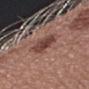Captured during whole-body skin photography for melanoma surveillance; the lesion was not biopsied.
A 15 mm crop from a total-body photograph taken for skin-cancer surveillance.
The subject is a female approximately 50 years of age.
On the left forearm.
The lesion's longest dimension is about 3 mm.
This is a white-light tile.